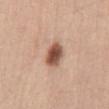Background:
The recorded lesion diameter is about 3.5 mm. Cropped from a whole-body photographic skin survey; the tile spans about 15 mm. Imaged with white-light lighting. Automated tile analysis of the lesion measured a footprint of about 7.5 mm² and an outline eccentricity of about 0.65 (0 = round, 1 = elongated). The analysis additionally found a border-irregularity index near 1.5/10, a within-lesion color-variation index near 5.5/10, and a peripheral color-asymmetry measure near 1.5. The analysis additionally found a classifier nevus-likeness of about 100/100 and a lesion-detection confidence of about 100/100. A female patient in their mid-30s. On the abdomen.